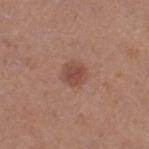The lesion was photographed on a routine skin check and not biopsied; there is no pathology result. Imaged with white-light lighting. A female patient, aged around 40. This image is a 15 mm lesion crop taken from a total-body photograph. The lesion is on the right thigh. The lesion's longest dimension is about 3 mm.